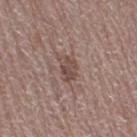Case summary:
- follow-up — total-body-photography surveillance lesion; no biopsy
- image — ~15 mm tile from a whole-body skin photo
- size — ~3 mm (longest diameter)
- illumination — white-light
- TBP lesion metrics — a lesion color around L≈47 a*≈18 b*≈21 in CIELAB; a lesion-detection confidence of about 100/100
- site — the right thigh
- subject — female, in their mid-60s